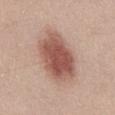Imaged during a routine full-body skin examination; the lesion was not biopsied and no histopathology is available. A male subject aged 23 to 27. The lesion's longest dimension is about 7 mm. On the abdomen. Cropped from a total-body skin-imaging series; the visible field is about 15 mm. This is a white-light tile. Automated image analysis of the tile measured roughly 14 lightness units darker than nearby skin and a lesion-to-skin contrast of about 9.5 (normalized; higher = more distinct). It also reported border irregularity of about 2 on a 0–10 scale and radial color variation of about 1.5. It also reported a detector confidence of about 100 out of 100 that the crop contains a lesion.